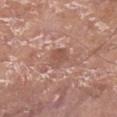Impression:
This lesion was catalogued during total-body skin photography and was not selected for biopsy.
Acquisition and patient details:
Located on the left lower leg. A roughly 15 mm field-of-view crop from a total-body skin photograph. The lesion's longest dimension is about 2.5 mm. The lesion-visualizer software estimated a footprint of about 4.5 mm² and an eccentricity of roughly 0.25. And it measured an average lesion color of about L≈52 a*≈22 b*≈27 (CIELAB), a lesion–skin lightness drop of about 8, and a lesion-to-skin contrast of about 6 (normalized; higher = more distinct). The analysis additionally found a border-irregularity index near 2/10, internal color variation of about 2 on a 0–10 scale, and peripheral color asymmetry of about 0.5. And it measured a nevus-likeness score of about 0/100. A male subject, aged around 70.